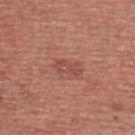Recorded during total-body skin imaging; not selected for excision or biopsy. From the chest. The total-body-photography lesion software estimated a footprint of about 4 mm², a shape eccentricity near 0.85, and a shape-asymmetry score of about 0.3 (0 = symmetric). It also reported border irregularity of about 4.5 on a 0–10 scale and a color-variation rating of about 2/10. The software also gave a nevus-likeness score of about 0/100 and a detector confidence of about 100 out of 100 that the crop contains a lesion. This image is a 15 mm lesion crop taken from a total-body photograph. The patient is a male aged around 45. The tile uses white-light illumination.